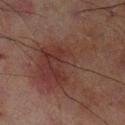The lesion was tiled from a total-body skin photograph and was not biopsied. Located on the left lower leg. A 15 mm crop from a total-body photograph taken for skin-cancer surveillance. A male patient about 70 years old.